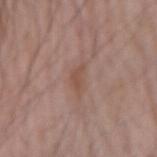This lesion was catalogued during total-body skin photography and was not selected for biopsy.
The recorded lesion diameter is about 3 mm.
Automated image analysis of the tile measured a lesion area of about 4 mm². It also reported about 6 CIELAB-L* units darker than the surrounding skin and a normalized lesion–skin contrast near 5.5. And it measured border irregularity of about 4 on a 0–10 scale and a color-variation rating of about 1.5/10.
Imaged with white-light lighting.
A male patient approximately 60 years of age.
The lesion is located on the right forearm.
Cropped from a whole-body photographic skin survey; the tile spans about 15 mm.